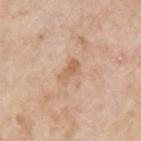The lesion was tiled from a total-body skin photograph and was not biopsied.
Measured at roughly 3 mm in maximum diameter.
A male subject, about 60 years old.
A 15 mm close-up tile from a total-body photography series done for melanoma screening.
On the left upper arm.